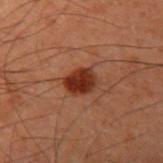Notes:
- workup: total-body-photography surveillance lesion; no biopsy
- image-analysis metrics: an area of roughly 7 mm², an outline eccentricity of about 0.65 (0 = round, 1 = elongated), and a symmetry-axis asymmetry near 0.2; an average lesion color of about L≈24 a*≈22 b*≈25 (CIELAB), a lesion–skin lightness drop of about 11, and a normalized border contrast of about 11.5; a border-irregularity index near 1.5/10, a within-lesion color-variation index near 3/10, and radial color variation of about 1; lesion-presence confidence of about 100/100
- tile lighting: cross-polarized illumination
- site: the left upper arm
- patient: male, aged approximately 60
- lesion size: about 3.5 mm
- acquisition: total-body-photography crop, ~15 mm field of view